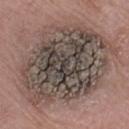lesion_size:
  long_diameter_mm_approx: 12.0
lighting: white-light
image:
  source: total-body photography crop
  field_of_view_mm: 15
patient:
  sex: male
  age_approx: 60
site: left forearm